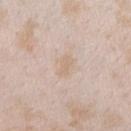  image:
    source: total-body photography crop
    field_of_view_mm: 15
  lighting: white-light
  patient:
    sex: female
    age_approx: 25
  site: right forearm
  lesion_size:
    long_diameter_mm_approx: 2.5